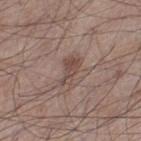This lesion was catalogued during total-body skin photography and was not selected for biopsy. This is a white-light tile. The subject is a male in their mid-50s. From the left thigh. Cropped from a total-body skin-imaging series; the visible field is about 15 mm. About 3.5 mm across.